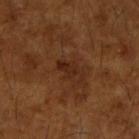| field | value |
|---|---|
| biopsy status | catalogued during a skin exam; not biopsied |
| lighting | cross-polarized illumination |
| patient | male, aged around 65 |
| location | the right upper arm |
| acquisition | ~15 mm tile from a whole-body skin photo |
| size | ~3.5 mm (longest diameter) |
| automated metrics | a mean CIELAB color near L≈26 a*≈21 b*≈29, about 7 CIELAB-L* units darker than the surrounding skin, and a normalized lesion–skin contrast near 7.5; border irregularity of about 3.5 on a 0–10 scale, a color-variation rating of about 2/10, and radial color variation of about 0.5; a classifier nevus-likeness of about 0/100 |